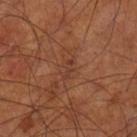biopsy status: catalogued during a skin exam; not biopsied.